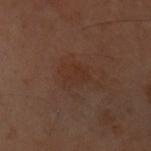Impression:
This lesion was catalogued during total-body skin photography and was not selected for biopsy.
Clinical summary:
A female subject, aged approximately 60. The lesion is located on the front of the torso. A close-up tile cropped from a whole-body skin photograph, about 15 mm across. Imaged with cross-polarized lighting.